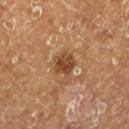workup: catalogued during a skin exam; not biopsied.